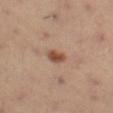Q: Was a biopsy performed?
A: catalogued during a skin exam; not biopsied
Q: What kind of image is this?
A: ~15 mm crop, total-body skin-cancer survey
Q: Patient demographics?
A: male, roughly 55 years of age
Q: Lesion location?
A: the left thigh